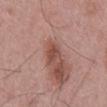This lesion was catalogued during total-body skin photography and was not selected for biopsy. A male subject roughly 55 years of age. From the back. Measured at roughly 2.5 mm in maximum diameter. Captured under white-light illumination. This image is a 15 mm lesion crop taken from a total-body photograph.